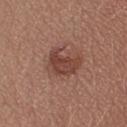Q: Is there a histopathology result?
A: no biopsy performed (imaged during a skin exam)
Q: Automated lesion metrics?
A: an average lesion color of about L≈42 a*≈23 b*≈26 (CIELAB), roughly 10 lightness units darker than nearby skin, and a lesion-to-skin contrast of about 7.5 (normalized; higher = more distinct); a border-irregularity rating of about 4.5/10 and radial color variation of about 1
Q: What is the lesion's diameter?
A: ≈4 mm
Q: What are the patient's age and sex?
A: female, roughly 35 years of age
Q: What is the imaging modality?
A: total-body-photography crop, ~15 mm field of view
Q: What is the anatomic site?
A: the head or neck
Q: What lighting was used for the tile?
A: white-light